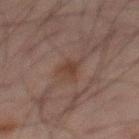Imaged during a routine full-body skin examination; the lesion was not biopsied and no histopathology is available.
Captured under cross-polarized illumination.
A 15 mm crop from a total-body photograph taken for skin-cancer surveillance.
About 3 mm across.
A male subject approximately 65 years of age.
From the back.